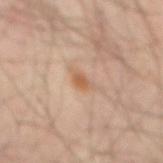Assessment:
Imaged during a routine full-body skin examination; the lesion was not biopsied and no histopathology is available.
Background:
A region of skin cropped from a whole-body photographic capture, roughly 15 mm wide. The lesion-visualizer software estimated an average lesion color of about L≈56 a*≈20 b*≈33 (CIELAB) and a lesion-to-skin contrast of about 7.5 (normalized; higher = more distinct). The software also gave a border-irregularity rating of about 3/10, a within-lesion color-variation index near 1/10, and peripheral color asymmetry of about 0.5. The software also gave an automated nevus-likeness rating near 50 out of 100 and a lesion-detection confidence of about 100/100. A male patient, about 65 years old. The lesion is located on the abdomen. The tile uses cross-polarized illumination. The recorded lesion diameter is about 2 mm.